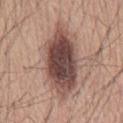follow-up — no biopsy performed (imaged during a skin exam) | lighting — white-light illumination | imaging modality — ~15 mm crop, total-body skin-cancer survey | location — the mid back | image-analysis metrics — a classifier nevus-likeness of about 90/100 and a lesion-detection confidence of about 100/100 | patient — male, aged 58 to 62 | size — about 9 mm.